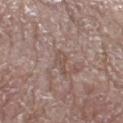Captured during whole-body skin photography for melanoma surveillance; the lesion was not biopsied. Captured under white-light illumination. From the left lower leg. Cropped from a total-body skin-imaging series; the visible field is about 15 mm. A female subject, in their mid- to late 80s or older. Automated image analysis of the tile measured an area of roughly 3.5 mm², an outline eccentricity of about 0.9 (0 = round, 1 = elongated), and two-axis asymmetry of about 0.35. The analysis additionally found a border-irregularity index near 4/10, internal color variation of about 0.5 on a 0–10 scale, and peripheral color asymmetry of about 0. The analysis additionally found a classifier nevus-likeness of about 0/100 and a detector confidence of about 60 out of 100 that the crop contains a lesion. About 3 mm across.